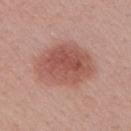Impression:
Imaged during a routine full-body skin examination; the lesion was not biopsied and no histopathology is available.
Acquisition and patient details:
Automated image analysis of the tile measured a lesion area of about 28 mm², a shape eccentricity near 0.6, and a shape-asymmetry score of about 0.15 (0 = symmetric). The software also gave a border-irregularity index near 1.5/10 and peripheral color asymmetry of about 1.5. The lesion is on the right upper arm. The tile uses white-light illumination. The lesion's longest dimension is about 7 mm. A female patient, approximately 55 years of age. A 15 mm crop from a total-body photograph taken for skin-cancer surveillance.